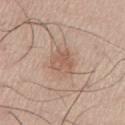The patient is a male in their mid- to late 50s. This is a white-light tile. Approximately 3 mm at its widest. A 15 mm crop from a total-body photograph taken for skin-cancer surveillance. The lesion is on the left lower leg. Automated image analysis of the tile measured an outline eccentricity of about 0.5 (0 = round, 1 = elongated) and a symmetry-axis asymmetry near 0.2. It also reported an average lesion color of about L≈58 a*≈19 b*≈28 (CIELAB) and about 8 CIELAB-L* units darker than the surrounding skin.